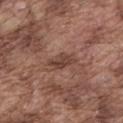Case summary:
- imaging modality · ~15 mm tile from a whole-body skin photo
- patient · male, in their mid- to late 70s
- TBP lesion metrics · a footprint of about 5 mm², an eccentricity of roughly 0.9, and two-axis asymmetry of about 0.35; about 8 CIELAB-L* units darker than the surrounding skin; a within-lesion color-variation index near 3.5/10 and peripheral color asymmetry of about 1.5
- diameter · ≈3.5 mm
- illumination · white-light illumination
- body site · the mid back